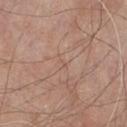Cropped from a total-body skin-imaging series; the visible field is about 15 mm. Longest diameter approximately 1 mm. The subject is a male approximately 80 years of age. Captured under white-light illumination. The lesion is on the chest.